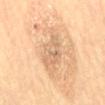Clinical impression: This lesion was catalogued during total-body skin photography and was not selected for biopsy. Acquisition and patient details: A male patient, about 70 years old. Imaged with cross-polarized lighting. An algorithmic analysis of the crop reported an area of roughly 14 mm², a shape eccentricity near 0.85, and a symmetry-axis asymmetry near 0.2. And it measured a lesion color around L≈68 a*≈17 b*≈36 in CIELAB, roughly 9 lightness units darker than nearby skin, and a normalized lesion–skin contrast near 5.5. The software also gave a border-irregularity index near 3.5/10, a within-lesion color-variation index near 4/10, and peripheral color asymmetry of about 1. The analysis additionally found a classifier nevus-likeness of about 0/100 and a detector confidence of about 95 out of 100 that the crop contains a lesion. Cropped from a total-body skin-imaging series; the visible field is about 15 mm. The lesion is on the front of the torso.